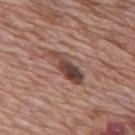No biopsy was performed on this lesion — it was imaged during a full skin examination and was not determined to be concerning. Automated tile analysis of the lesion measured a lesion area of about 7.5 mm². It also reported a mean CIELAB color near L≈43 a*≈20 b*≈23, roughly 14 lightness units darker than nearby skin, and a normalized lesion–skin contrast near 10.5. The analysis additionally found border irregularity of about 4 on a 0–10 scale, internal color variation of about 6.5 on a 0–10 scale, and a peripheral color-asymmetry measure near 2. Approximately 5 mm at its widest. A close-up tile cropped from a whole-body skin photograph, about 15 mm across. Located on the mid back. A male patient roughly 70 years of age. This is a white-light tile.